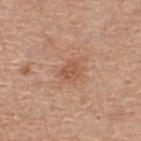{
  "biopsy_status": "not biopsied; imaged during a skin examination",
  "site": "upper back",
  "image": {
    "source": "total-body photography crop",
    "field_of_view_mm": 15
  },
  "lighting": "white-light",
  "patient": {
    "sex": "male",
    "age_approx": 55
  }
}